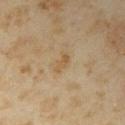Captured during whole-body skin photography for melanoma surveillance; the lesion was not biopsied. The tile uses cross-polarized illumination. A 15 mm crop from a total-body photograph taken for skin-cancer surveillance. The lesion is on the right upper arm. Approximately 2.5 mm at its widest. A female subject in their mid-30s.